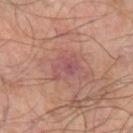Recorded during total-body skin imaging; not selected for excision or biopsy. Cropped from a whole-body photographic skin survey; the tile spans about 15 mm. Automated image analysis of the tile measured a lesion area of about 5.5 mm², a shape eccentricity near 0.75, and two-axis asymmetry of about 0.4. Imaged with cross-polarized lighting. The recorded lesion diameter is about 3.5 mm. On the left forearm. A male patient, in their mid-60s.